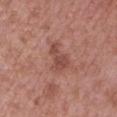| field | value |
|---|---|
| workup | no biopsy performed (imaged during a skin exam) |
| location | the arm |
| size | ≈4 mm |
| patient | male, aged around 50 |
| acquisition | total-body-photography crop, ~15 mm field of view |
| illumination | white-light illumination |